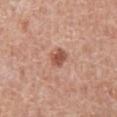Notes:
* notes · imaged on a skin check; not biopsied
* lighting · white-light illumination
* size · ~2.5 mm (longest diameter)
* anatomic site · the abdomen
* patient · male, in their mid-70s
* image-analysis metrics · a lesion color around L≈53 a*≈25 b*≈29 in CIELAB, a lesion–skin lightness drop of about 12, and a lesion-to-skin contrast of about 8 (normalized; higher = more distinct); a border-irregularity rating of about 2/10, internal color variation of about 2 on a 0–10 scale, and a peripheral color-asymmetry measure near 0.5; an automated nevus-likeness rating near 85 out of 100 and a detector confidence of about 100 out of 100 that the crop contains a lesion
* imaging modality · 15 mm crop, total-body photography